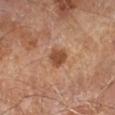Notes:
• notes · no biopsy performed (imaged during a skin exam)
• automated lesion analysis · an outline eccentricity of about 0.5 (0 = round, 1 = elongated); a border-irregularity index near 2.5/10, a within-lesion color-variation index near 2/10, and peripheral color asymmetry of about 0.5
• anatomic site · the right forearm
• illumination · cross-polarized illumination
• patient · male, aged around 65
• diameter · ≈3 mm
• image source · 15 mm crop, total-body photography